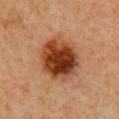The lesion was tiled from a total-body skin photograph and was not biopsied.
A female patient about 40 years old.
About 5.5 mm across.
A roughly 15 mm field-of-view crop from a total-body skin photograph.
Automated tile analysis of the lesion measured a within-lesion color-variation index near 7/10 and a peripheral color-asymmetry measure near 2.
On the chest.
This is a cross-polarized tile.